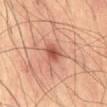biopsy status: total-body-photography surveillance lesion; no biopsy | size: ≈2.5 mm | subject: male, approximately 65 years of age | automated lesion analysis: a lesion area of about 4 mm² and a shape eccentricity near 0.6; about 11 CIELAB-L* units darker than the surrounding skin; a border-irregularity rating of about 2/10, internal color variation of about 3 on a 0–10 scale, and a peripheral color-asymmetry measure near 0.5 | acquisition: 15 mm crop, total-body photography | location: the abdomen.